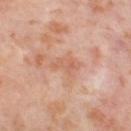<tbp_lesion>
  <biopsy_status>not biopsied; imaged during a skin examination</biopsy_status>
  <patient>
    <sex>female</sex>
    <age_approx>55</age_approx>
  </patient>
  <site>right thigh</site>
  <image>
    <source>total-body photography crop</source>
    <field_of_view_mm>15</field_of_view_mm>
  </image>
  <lesion_size>
    <long_diameter_mm_approx>3.5</long_diameter_mm_approx>
  </lesion_size>
  <automated_metrics>
    <cielab_L>62</cielab_L>
    <cielab_a>23</cielab_a>
    <cielab_b>32</cielab_b>
    <vs_skin_darker_L>7.0</vs_skin_darker_L>
    <vs_skin_contrast_norm>5.0</vs_skin_contrast_norm>
    <color_variation_0_10>2.5</color_variation_0_10>
    <peripheral_color_asymmetry>0.5</peripheral_color_asymmetry>
  </automated_metrics>
</tbp_lesion>